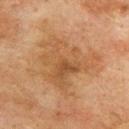Background: The recorded lesion diameter is about 7.5 mm. The total-body-photography lesion software estimated a lesion area of about 27 mm² and a shape-asymmetry score of about 0.45 (0 = symmetric). And it measured roughly 6 lightness units darker than nearby skin and a normalized border contrast of about 5.5. It also reported a border-irregularity index near 8.5/10, a color-variation rating of about 4.5/10, and a peripheral color-asymmetry measure near 1.5. And it measured a nevus-likeness score of about 0/100 and a detector confidence of about 100 out of 100 that the crop contains a lesion. This is a cross-polarized tile. The lesion is located on the upper back. Cropped from a whole-body photographic skin survey; the tile spans about 15 mm. A male subject aged around 75.